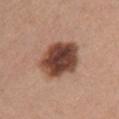| feature | finding |
|---|---|
| biopsy status | imaged on a skin check; not biopsied |
| anatomic site | the chest |
| acquisition | ~15 mm crop, total-body skin-cancer survey |
| TBP lesion metrics | a footprint of about 20 mm², an eccentricity of roughly 0.65, and two-axis asymmetry of about 0.15; a mean CIELAB color near L≈44 a*≈21 b*≈27 and a normalized border contrast of about 13.5; an automated nevus-likeness rating near 60 out of 100 and a lesion-detection confidence of about 100/100 |
| patient | female, aged 43–47 |
| lesion diameter | ≈6 mm |
| lighting | white-light |